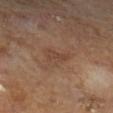Clinical impression: Part of a total-body skin-imaging series; this lesion was reviewed on a skin check and was not flagged for biopsy. Acquisition and patient details: The tile uses cross-polarized illumination. A male subject roughly 65 years of age. A close-up tile cropped from a whole-body skin photograph, about 15 mm across. Approximately 3 mm at its widest.